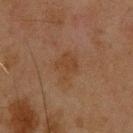| field | value |
|---|---|
| follow-up | imaged on a skin check; not biopsied |
| patient | male, about 45 years old |
| lighting | cross-polarized illumination |
| imaging modality | ~15 mm crop, total-body skin-cancer survey |
| site | the back |
| lesion size | ~3 mm (longest diameter) |